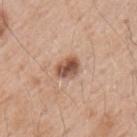Case summary:
* biopsy status — total-body-photography surveillance lesion; no biopsy
* image-analysis metrics — an area of roughly 5.5 mm², a shape eccentricity near 0.6, and a symmetry-axis asymmetry near 0.15; an average lesion color of about L≈53 a*≈21 b*≈29 (CIELAB), roughly 15 lightness units darker than nearby skin, and a lesion-to-skin contrast of about 10 (normalized; higher = more distinct); a border-irregularity index near 1.5/10, internal color variation of about 5.5 on a 0–10 scale, and radial color variation of about 2; a nevus-likeness score of about 70/100 and a lesion-detection confidence of about 100/100
* illumination — white-light
* image — ~15 mm crop, total-body skin-cancer survey
* lesion diameter — ≈3 mm
* subject — male, aged 48 to 52
* site — the left upper arm